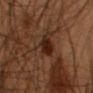<case>
<biopsy_status>not biopsied; imaged during a skin examination</biopsy_status>
<lighting>cross-polarized</lighting>
<patient>
  <sex>male</sex>
  <age_approx>55</age_approx>
</patient>
<image>
  <source>total-body photography crop</source>
  <field_of_view_mm>15</field_of_view_mm>
</image>
<lesion_size>
  <long_diameter_mm_approx>3.0</long_diameter_mm_approx>
</lesion_size>
<site>right forearm</site>
</case>